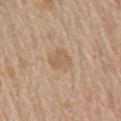Q: Was a biopsy performed?
A: total-body-photography surveillance lesion; no biopsy
Q: What is the lesion's diameter?
A: about 3.5 mm
Q: What is the anatomic site?
A: the lower back
Q: How was this image acquired?
A: ~15 mm crop, total-body skin-cancer survey
Q: Who is the patient?
A: male, aged around 70
Q: How was the tile lit?
A: white-light